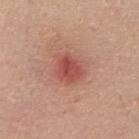Part of a total-body skin-imaging series; this lesion was reviewed on a skin check and was not flagged for biopsy.
Approximately 3 mm at its widest.
The lesion is on the upper back.
A male patient roughly 30 years of age.
Cropped from a whole-body photographic skin survey; the tile spans about 15 mm.
Captured under white-light illumination.
An algorithmic analysis of the crop reported an eccentricity of roughly 0.45 and a shape-asymmetry score of about 0.2 (0 = symmetric). The software also gave a lesion color around L≈51 a*≈30 b*≈27 in CIELAB. The analysis additionally found a border-irregularity index near 2/10, a color-variation rating of about 3.5/10, and peripheral color asymmetry of about 1.5. It also reported a nevus-likeness score of about 30/100.